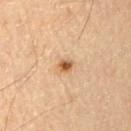workup: total-body-photography surveillance lesion; no biopsy | size: ≈2 mm | illumination: cross-polarized illumination | patient: male, roughly 55 years of age | image: ~15 mm tile from a whole-body skin photo | site: the arm.